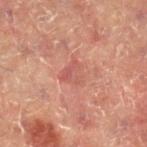Impression:
The lesion was photographed on a routine skin check and not biopsied; there is no pathology result.